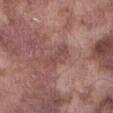Background: Captured under white-light illumination. Measured at roughly 4 mm in maximum diameter. Located on the front of the torso. A roughly 15 mm field-of-view crop from a total-body skin photograph. A male subject, approximately 75 years of age.